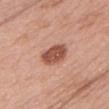Recorded during total-body skin imaging; not selected for excision or biopsy.
The lesion is on the head or neck.
Measured at roughly 4 mm in maximum diameter.
A female patient aged around 60.
A 15 mm crop from a total-body photograph taken for skin-cancer surveillance.
An algorithmic analysis of the crop reported an outline eccentricity of about 0.75 (0 = round, 1 = elongated). And it measured a lesion color around L≈52 a*≈25 b*≈29 in CIELAB, about 15 CIELAB-L* units darker than the surrounding skin, and a normalized lesion–skin contrast near 9.5. And it measured an automated nevus-likeness rating near 80 out of 100 and lesion-presence confidence of about 100/100.
Imaged with white-light lighting.